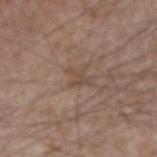follow-up = imaged on a skin check; not biopsied | imaging modality = 15 mm crop, total-body photography | patient = male, approximately 75 years of age | body site = the right forearm.